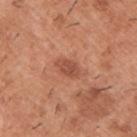Clinical impression:
This lesion was catalogued during total-body skin photography and was not selected for biopsy.
Image and clinical context:
Cropped from a whole-body photographic skin survey; the tile spans about 15 mm. The patient is a male approximately 40 years of age. The lesion is on the back. Imaged with white-light lighting.